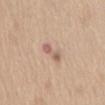Notes:
- subject — male, aged around 70
- acquisition — total-body-photography crop, ~15 mm field of view
- body site — the abdomen